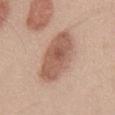The lesion was tiled from a total-body skin photograph and was not biopsied. Longest diameter approximately 7 mm. The tile uses white-light illumination. A 15 mm crop from a total-body photograph taken for skin-cancer surveillance. A male subject about 50 years old. The lesion is on the chest.